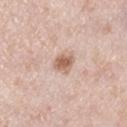Imaged during a routine full-body skin examination; the lesion was not biopsied and no histopathology is available.
Longest diameter approximately 2.5 mm.
A 15 mm crop from a total-body photograph taken for skin-cancer surveillance.
The tile uses white-light illumination.
The patient is a female approximately 40 years of age.
The lesion is located on the right lower leg.